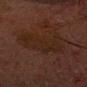Captured during whole-body skin photography for melanoma surveillance; the lesion was not biopsied.